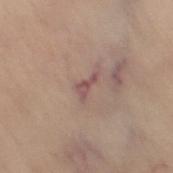<record>
<biopsy_status>not biopsied; imaged during a skin examination</biopsy_status>
<image>
  <source>total-body photography crop</source>
  <field_of_view_mm>15</field_of_view_mm>
</image>
<patient>
  <sex>female</sex>
  <age_approx>60</age_approx>
</patient>
<site>left leg</site>
<lighting>cross-polarized</lighting>
<lesion_size>
  <long_diameter_mm_approx>3.0</long_diameter_mm_approx>
</lesion_size>
</record>